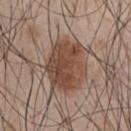Image and clinical context: The recorded lesion diameter is about 6 mm. A male patient aged 43–47. This is a white-light tile. From the chest. A 15 mm crop from a total-body photograph taken for skin-cancer surveillance. Automated tile analysis of the lesion measured a symmetry-axis asymmetry near 0.15. It also reported an average lesion color of about L≈45 a*≈18 b*≈27 (CIELAB) and a normalized lesion–skin contrast near 9.5. And it measured border irregularity of about 2 on a 0–10 scale, a within-lesion color-variation index near 4.5/10, and peripheral color asymmetry of about 1.5.